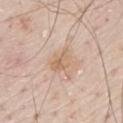Q: Was a biopsy performed?
A: imaged on a skin check; not biopsied
Q: How was the tile lit?
A: white-light
Q: What are the patient's age and sex?
A: male, aged 78–82
Q: Lesion location?
A: the mid back
Q: What kind of image is this?
A: ~15 mm tile from a whole-body skin photo
Q: What did automated image analysis measure?
A: a lesion area of about 5 mm² and a symmetry-axis asymmetry near 0.35; a border-irregularity rating of about 4/10, internal color variation of about 4 on a 0–10 scale, and a peripheral color-asymmetry measure near 1.5